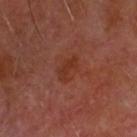{"biopsy_status": "not biopsied; imaged during a skin examination", "patient": {"sex": "male", "age_approx": 60}, "lighting": "cross-polarized", "automated_metrics": {"lesion_detection_confidence_0_100": 100}, "lesion_size": {"long_diameter_mm_approx": 3.0}, "image": {"source": "total-body photography crop", "field_of_view_mm": 15}, "site": "head or neck"}